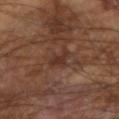biopsy_status: not biopsied; imaged during a skin examination
patient:
  sex: male
  age_approx: 65
automated_metrics:
  shape_asymmetry: 0.5
  color_variation_0_10: 1.0
  peripheral_color_asymmetry: 0.5
image:
  source: total-body photography crop
  field_of_view_mm: 15
site: left arm